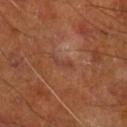The lesion was tiled from a total-body skin photograph and was not biopsied.
A 15 mm crop from a total-body photograph taken for skin-cancer surveillance.
On the right lower leg.
Captured under cross-polarized illumination.
The lesion's longest dimension is about 2.5 mm.
The total-body-photography lesion software estimated an average lesion color of about L≈39 a*≈23 b*≈28 (CIELAB), a lesion–skin lightness drop of about 5, and a lesion-to-skin contrast of about 4.5 (normalized; higher = more distinct). And it measured a nevus-likeness score of about 0/100.
A male patient roughly 65 years of age.